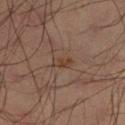biopsy status — total-body-photography surveillance lesion; no biopsy | illumination — cross-polarized | subject — male, aged approximately 35 | location — the left lower leg | image source — total-body-photography crop, ~15 mm field of view | diameter — ≈3 mm.